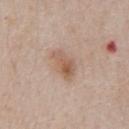patient — male, aged 58 to 62
lesion diameter — ≈4 mm
lighting — white-light illumination
automated metrics — an area of roughly 7 mm², an outline eccentricity of about 0.8 (0 = round, 1 = elongated), and a symmetry-axis asymmetry near 0.3; border irregularity of about 3 on a 0–10 scale, a color-variation rating of about 6/10, and peripheral color asymmetry of about 2; a nevus-likeness score of about 50/100 and a detector confidence of about 100 out of 100 that the crop contains a lesion
imaging modality — total-body-photography crop, ~15 mm field of view
site — the chest